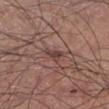This lesion was catalogued during total-body skin photography and was not selected for biopsy.
A male patient aged 43–47.
A close-up tile cropped from a whole-body skin photograph, about 15 mm across.
About 2.5 mm across.
The lesion is on the left lower leg.
An algorithmic analysis of the crop reported a shape eccentricity near 0.85 and two-axis asymmetry of about 0.5. The analysis additionally found an average lesion color of about L≈41 a*≈19 b*≈21 (CIELAB), roughly 9 lightness units darker than nearby skin, and a lesion-to-skin contrast of about 7.5 (normalized; higher = more distinct). It also reported border irregularity of about 5 on a 0–10 scale and a within-lesion color-variation index near 0.5/10.
Captured under white-light illumination.